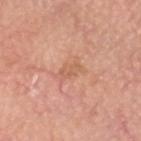About 3.5 mm across.
Automated image analysis of the tile measured a mean CIELAB color near L≈61 a*≈24 b*≈32 and a lesion-to-skin contrast of about 4.5 (normalized; higher = more distinct).
From the head or neck.
A lesion tile, about 15 mm wide, cut from a 3D total-body photograph.
The tile uses white-light illumination.
A male subject about 65 years old.